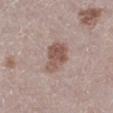Case summary:
– follow-up — total-body-photography surveillance lesion; no biopsy
– patient — female, aged approximately 50
– lesion size — about 4 mm
– automated lesion analysis — an area of roughly 8.5 mm² and two-axis asymmetry of about 0.3; border irregularity of about 2.5 on a 0–10 scale, a within-lesion color-variation index near 3.5/10, and a peripheral color-asymmetry measure near 1
– image — ~15 mm crop, total-body skin-cancer survey
– body site — the leg
– tile lighting — white-light illumination